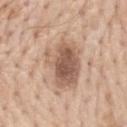Clinical impression:
No biopsy was performed on this lesion — it was imaged during a full skin examination and was not determined to be concerning.
Background:
Cropped from a whole-body photographic skin survey; the tile spans about 15 mm. A male patient, about 70 years old. The lesion is located on the mid back. About 7.5 mm across. Imaged with white-light lighting. Automated tile analysis of the lesion measured an average lesion color of about L≈60 a*≈17 b*≈29 (CIELAB) and a normalized lesion–skin contrast near 7. The software also gave a classifier nevus-likeness of about 85/100 and lesion-presence confidence of about 100/100.